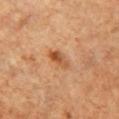Q: Was this lesion biopsied?
A: catalogued during a skin exam; not biopsied
Q: What is the imaging modality?
A: ~15 mm tile from a whole-body skin photo
Q: Patient demographics?
A: female, approximately 60 years of age
Q: Where on the body is the lesion?
A: the chest